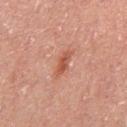The lesion was tiled from a total-body skin photograph and was not biopsied. Captured under cross-polarized illumination. From the back. A close-up tile cropped from a whole-body skin photograph, about 15 mm across. The lesion-visualizer software estimated a footprint of about 4 mm², an outline eccentricity of about 0.9 (0 = round, 1 = elongated), and a symmetry-axis asymmetry near 0.3. The analysis additionally found a lesion color around L≈40 a*≈21 b*≈25 in CIELAB, roughly 8 lightness units darker than nearby skin, and a normalized border contrast of about 7. The software also gave border irregularity of about 3.5 on a 0–10 scale, internal color variation of about 2.5 on a 0–10 scale, and radial color variation of about 1. It also reported a classifier nevus-likeness of about 10/100 and lesion-presence confidence of about 100/100. A male patient roughly 60 years of age. Measured at roughly 3.5 mm in maximum diameter.